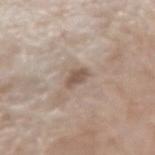Assessment:
Part of a total-body skin-imaging series; this lesion was reviewed on a skin check and was not flagged for biopsy.
Acquisition and patient details:
A female subject, aged 73–77. Approximately 3 mm at its widest. This is a white-light tile. A 15 mm close-up tile from a total-body photography series done for melanoma screening. The lesion-visualizer software estimated an area of roughly 3.5 mm², an eccentricity of roughly 0.8, and a shape-asymmetry score of about 0.25 (0 = symmetric). It also reported a lesion color around L≈53 a*≈14 b*≈25 in CIELAB, about 11 CIELAB-L* units darker than the surrounding skin, and a normalized border contrast of about 7.5. It also reported border irregularity of about 2.5 on a 0–10 scale, a color-variation rating of about 1/10, and a peripheral color-asymmetry measure near 0.5. The lesion is located on the right forearm.